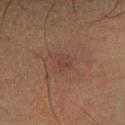notes=no biopsy performed (imaged during a skin exam)
location=the right lower leg
image source=15 mm crop, total-body photography
patient=male, roughly 65 years of age